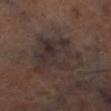Recorded during total-body skin imaging; not selected for excision or biopsy.
The lesion is on the left lower leg.
Automated image analysis of the tile measured border irregularity of about 4.5 on a 0–10 scale and a color-variation rating of about 5.5/10. The software also gave an automated nevus-likeness rating near 0 out of 100 and a lesion-detection confidence of about 75/100.
Imaged with cross-polarized lighting.
A 15 mm close-up extracted from a 3D total-body photography capture.
The lesion's longest dimension is about 6.5 mm.